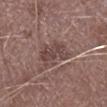Findings:
• notes — total-body-photography surveillance lesion; no biopsy
• patient — male, aged 73 to 77
• anatomic site — the right lower leg
• image — total-body-photography crop, ~15 mm field of view